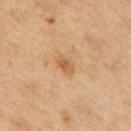Case summary:
• follow-up · imaged on a skin check; not biopsied
• image-analysis metrics · a footprint of about 3 mm² and an outline eccentricity of about 0.85 (0 = round, 1 = elongated); a detector confidence of about 100 out of 100 that the crop contains a lesion
• patient · male, roughly 70 years of age
• acquisition · ~15 mm crop, total-body skin-cancer survey
• lesion diameter · ≈2.5 mm
• body site · the mid back
• illumination · cross-polarized illumination